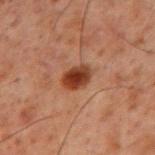Notes:
* biopsy status · no biopsy performed (imaged during a skin exam)
* location · the mid back
* subject · male, roughly 60 years of age
* image · ~15 mm crop, total-body skin-cancer survey